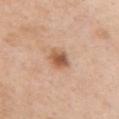image:
  source: total-body photography crop
  field_of_view_mm: 15
patient:
  sex: female
  age_approx: 55
site: chest